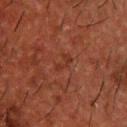<case>
  <biopsy_status>not biopsied; imaged during a skin examination</biopsy_status>
  <lesion_size>
    <long_diameter_mm_approx>2.5</long_diameter_mm_approx>
  </lesion_size>
  <lighting>cross-polarized</lighting>
  <patient>
    <sex>male</sex>
    <age_approx>50</age_approx>
  </patient>
  <image>
    <source>total-body photography crop</source>
    <field_of_view_mm>15</field_of_view_mm>
  </image>
  <site>upper back</site>
  <automated_metrics>
    <cielab_L>26</cielab_L>
    <cielab_a>22</cielab_a>
    <cielab_b>25</cielab_b>
    <vs_skin_contrast_norm>5.0</vs_skin_contrast_norm>
    <border_irregularity_0_10>5.5</border_irregularity_0_10>
    <color_variation_0_10>0.0</color_variation_0_10>
    <peripheral_color_asymmetry>0.0</peripheral_color_asymmetry>
    <nevus_likeness_0_100>0</nevus_likeness_0_100>
    <lesion_detection_confidence_0_100>100</lesion_detection_confidence_0_100>
  </automated_metrics>
</case>